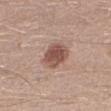subject = male, about 30 years old
anatomic site = the left lower leg
tile lighting = white-light
TBP lesion metrics = a mean CIELAB color near L≈52 a*≈20 b*≈25, about 13 CIELAB-L* units darker than the surrounding skin, and a normalized border contrast of about 9; a nevus-likeness score of about 90/100 and a lesion-detection confidence of about 100/100
image source = ~15 mm tile from a whole-body skin photo
lesion diameter = ≈3.5 mm